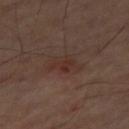Findings:
• workup — imaged on a skin check; not biopsied
• imaging modality — 15 mm crop, total-body photography
• size — ~4 mm (longest diameter)
• anatomic site — the right thigh